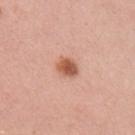  biopsy_status: not biopsied; imaged during a skin examination
  site: arm
  automated_metrics:
    eccentricity: 0.6
    shape_asymmetry: 0.2
    vs_skin_darker_L: 14.0
    vs_skin_contrast_norm: 9.5
    border_irregularity_0_10: 1.5
    color_variation_0_10: 3.5
    nevus_likeness_0_100: 100
  patient:
    sex: female
    age_approx: 40
  lighting: white-light
  image:
    source: total-body photography crop
    field_of_view_mm: 15
  lesion_size:
    long_diameter_mm_approx: 2.5